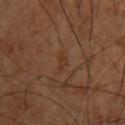This is a cross-polarized tile.
A male subject approximately 60 years of age.
A lesion tile, about 15 mm wide, cut from a 3D total-body photograph.
Longest diameter approximately 2.5 mm.
From the upper back.